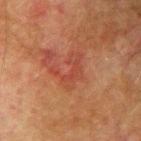Case summary:
* follow-up — catalogued during a skin exam; not biopsied
* patient — male, in their mid- to late 70s
* body site — the right upper arm
* automated lesion analysis — a mean CIELAB color near L≈39 a*≈26 b*≈28, about 6 CIELAB-L* units darker than the surrounding skin, and a lesion-to-skin contrast of about 5 (normalized; higher = more distinct); a border-irregularity index near 9/10; a nevus-likeness score of about 0/100
* size — ~4 mm (longest diameter)
* imaging modality — 15 mm crop, total-body photography
* lighting — cross-polarized illumination